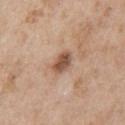Notes:
• workup: no biopsy performed (imaged during a skin exam)
• site: the chest
• patient: male, aged 63 to 67
• acquisition: ~15 mm crop, total-body skin-cancer survey
• lesion diameter: about 3 mm